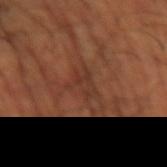biopsy_status: not biopsied; imaged during a skin examination
lesion_size:
  long_diameter_mm_approx: 3.5
site: left thigh
patient:
  sex: male
  age_approx: 65
image:
  source: total-body photography crop
  field_of_view_mm: 15